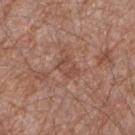<record>
  <biopsy_status>not biopsied; imaged during a skin examination</biopsy_status>
  <site>right forearm</site>
  <patient>
    <sex>male</sex>
    <age_approx>55</age_approx>
  </patient>
  <image>
    <source>total-body photography crop</source>
    <field_of_view_mm>15</field_of_view_mm>
  </image>
</record>